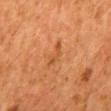Q: Was this lesion biopsied?
A: no biopsy performed (imaged during a skin exam)
Q: Patient demographics?
A: female, about 50 years old
Q: What is the lesion's diameter?
A: ~3.5 mm (longest diameter)
Q: Lesion location?
A: the mid back
Q: What is the imaging modality?
A: 15 mm crop, total-body photography